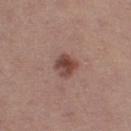{"biopsy_status": "not biopsied; imaged during a skin examination", "site": "left thigh", "automated_metrics": {"color_variation_0_10": 5.0, "peripheral_color_asymmetry": 1.5, "nevus_likeness_0_100": 90, "lesion_detection_confidence_0_100": 100}, "lighting": "white-light", "patient": {"sex": "female", "age_approx": 20}, "image": {"source": "total-body photography crop", "field_of_view_mm": 15}}